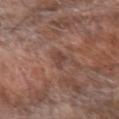follow-up = imaged on a skin check; not biopsied
image = 15 mm crop, total-body photography
patient = male, aged 68–72
body site = the left forearm